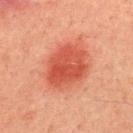Findings:
- notes — no biopsy performed (imaged during a skin exam)
- patient — male, about 45 years old
- imaging modality — ~15 mm crop, total-body skin-cancer survey
- location — the mid back